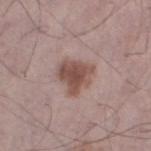Recorded during total-body skin imaging; not selected for excision or biopsy. The lesion-visualizer software estimated a peripheral color-asymmetry measure near 1. The software also gave a nevus-likeness score of about 75/100. A male patient, about 70 years old. Imaged with white-light lighting. The lesion is on the left thigh. The lesion's longest dimension is about 4 mm. A 15 mm close-up tile from a total-body photography series done for melanoma screening.